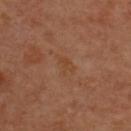<lesion>
<biopsy_status>not biopsied; imaged during a skin examination</biopsy_status>
<patient>
  <sex>male</sex>
  <age_approx>65</age_approx>
</patient>
<site>back</site>
<image>
  <source>total-body photography crop</source>
  <field_of_view_mm>15</field_of_view_mm>
</image>
</lesion>